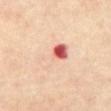Imaged during a routine full-body skin examination; the lesion was not biopsied and no histopathology is available.
A male patient, aged 58–62.
Captured under cross-polarized illumination.
Measured at roughly 4.5 mm in maximum diameter.
An algorithmic analysis of the crop reported a mean CIELAB color near L≈67 a*≈23 b*≈29, a lesion–skin lightness drop of about 10, and a normalized border contrast of about 6. The software also gave a border-irregularity rating of about 3.5/10, a color-variation rating of about 10/10, and radial color variation of about 10.5.
From the abdomen.
A roughly 15 mm field-of-view crop from a total-body skin photograph.